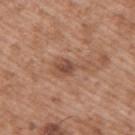image:
  source: total-body photography crop
  field_of_view_mm: 15
lighting: white-light
patient:
  sex: male
  age_approx: 50
lesion_size:
  long_diameter_mm_approx: 4.5
site: upper back